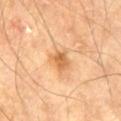notes: total-body-photography surveillance lesion; no biopsy
image source: ~15 mm crop, total-body skin-cancer survey
lesion size: ~2.5 mm (longest diameter)
subject: male, about 70 years old
automated lesion analysis: a border-irregularity rating of about 2.5/10, internal color variation of about 3.5 on a 0–10 scale, and peripheral color asymmetry of about 1; an automated nevus-likeness rating near 15 out of 100 and lesion-presence confidence of about 100/100
illumination: cross-polarized illumination
body site: the left thigh